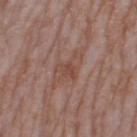Clinical impression: This lesion was catalogued during total-body skin photography and was not selected for biopsy. Background: Captured under white-light illumination. Cropped from a whole-body photographic skin survey; the tile spans about 15 mm. The subject is a female aged around 60. Approximately 4.5 mm at its widest. On the left thigh. An algorithmic analysis of the crop reported a detector confidence of about 90 out of 100 that the crop contains a lesion.